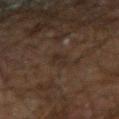No biopsy was performed on this lesion — it was imaged during a full skin examination and was not determined to be concerning.
An algorithmic analysis of the crop reported an area of roughly 3 mm², an eccentricity of roughly 0.85, and two-axis asymmetry of about 0.3. And it measured an average lesion color of about L≈21 a*≈10 b*≈17 (CIELAB), a lesion–skin lightness drop of about 4, and a lesion-to-skin contrast of about 5.5 (normalized; higher = more distinct). The analysis additionally found a border-irregularity index near 3/10, a color-variation rating of about 1/10, and a peripheral color-asymmetry measure near 0.5. It also reported a nevus-likeness score of about 0/100 and lesion-presence confidence of about 75/100.
A close-up tile cropped from a whole-body skin photograph, about 15 mm across.
The tile uses cross-polarized illumination.
The lesion is located on the right forearm.
A male subject aged approximately 70.
Measured at roughly 2.5 mm in maximum diameter.